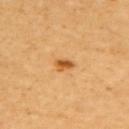Image and clinical context: A 15 mm close-up extracted from a 3D total-body photography capture. An algorithmic analysis of the crop reported an outline eccentricity of about 0.8 (0 = round, 1 = elongated). The software also gave about 14 CIELAB-L* units darker than the surrounding skin and a normalized border contrast of about 9. The software also gave border irregularity of about 2 on a 0–10 scale, internal color variation of about 2.5 on a 0–10 scale, and a peripheral color-asymmetry measure near 0.5. And it measured a classifier nevus-likeness of about 95/100 and a detector confidence of about 100 out of 100 that the crop contains a lesion. Located on the left arm. A female patient aged approximately 60. Imaged with cross-polarized lighting. The lesion's longest dimension is about 2.5 mm.